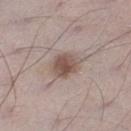Impression:
Imaged during a routine full-body skin examination; the lesion was not biopsied and no histopathology is available.
Image and clinical context:
Measured at roughly 3.5 mm in maximum diameter. The subject is a male aged 68 to 72. The lesion is on the left lower leg. The tile uses white-light illumination. A lesion tile, about 15 mm wide, cut from a 3D total-body photograph.